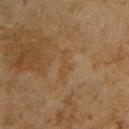<record>
  <biopsy_status>not biopsied; imaged during a skin examination</biopsy_status>
  <patient>
    <sex>male</sex>
    <age_approx>45</age_approx>
  </patient>
  <site>back</site>
  <image>
    <source>total-body photography crop</source>
    <field_of_view_mm>15</field_of_view_mm>
  </image>
  <lighting>cross-polarized</lighting>
</record>